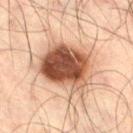follow-up: catalogued during a skin exam; not biopsied
patient: male, aged around 50
image source: ~15 mm crop, total-body skin-cancer survey
anatomic site: the right thigh